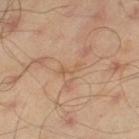Impression: This lesion was catalogued during total-body skin photography and was not selected for biopsy. Context: On the left thigh. The patient is a male approximately 45 years of age. A lesion tile, about 15 mm wide, cut from a 3D total-body photograph.